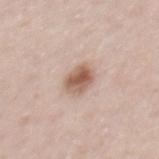The lesion was tiled from a total-body skin photograph and was not biopsied.
An algorithmic analysis of the crop reported an average lesion color of about L≈58 a*≈19 b*≈27 (CIELAB) and about 14 CIELAB-L* units darker than the surrounding skin.
From the mid back.
The lesion's longest dimension is about 3 mm.
This image is a 15 mm lesion crop taken from a total-body photograph.
Imaged with white-light lighting.
A male patient aged 38 to 42.